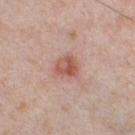Imaged during a routine full-body skin examination; the lesion was not biopsied and no histopathology is available. About 2.5 mm across. Located on the chest. A region of skin cropped from a whole-body photographic capture, roughly 15 mm wide. Imaged with white-light lighting. The patient is a male about 40 years old.